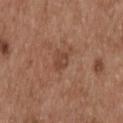<lesion>
  <biopsy_status>not biopsied; imaged during a skin examination</biopsy_status>
  <image>
    <source>total-body photography crop</source>
    <field_of_view_mm>15</field_of_view_mm>
  </image>
  <patient>
    <sex>male</sex>
    <age_approx>55</age_approx>
  </patient>
  <lesion_size>
    <long_diameter_mm_approx>2.5</long_diameter_mm_approx>
  </lesion_size>
  <lighting>white-light</lighting>
  <site>upper back</site>
</lesion>